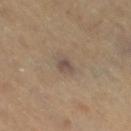Findings:
* follow-up: total-body-photography surveillance lesion; no biopsy